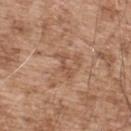Impression: Captured during whole-body skin photography for melanoma surveillance; the lesion was not biopsied. Clinical summary: The lesion is on the upper back. A roughly 15 mm field-of-view crop from a total-body skin photograph. The subject is a male aged approximately 55.